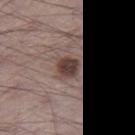{
  "biopsy_status": "not biopsied; imaged during a skin examination",
  "site": "right thigh",
  "image": {
    "source": "total-body photography crop",
    "field_of_view_mm": 15
  },
  "patient": {
    "sex": "male",
    "age_approx": 70
  },
  "automated_metrics": {
    "cielab_L": 40,
    "cielab_a": 16,
    "cielab_b": 20,
    "vs_skin_darker_L": 13.0,
    "vs_skin_contrast_norm": 10.5,
    "nevus_likeness_0_100": 90
  },
  "lighting": "white-light",
  "lesion_size": {
    "long_diameter_mm_approx": 2.5
  }
}